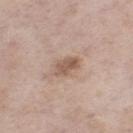{"biopsy_status": "not biopsied; imaged during a skin examination", "lighting": "white-light", "patient": {"sex": "female", "age_approx": 55}, "image": {"source": "total-body photography crop", "field_of_view_mm": 15}, "site": "left thigh", "automated_metrics": {"eccentricity": 0.85, "shape_asymmetry": 0.2, "cielab_L": 55, "cielab_a": 17, "cielab_b": 27, "vs_skin_darker_L": 11.0, "vs_skin_contrast_norm": 7.5, "border_irregularity_0_10": 2.0, "color_variation_0_10": 2.5, "peripheral_color_asymmetry": 1.0, "nevus_likeness_0_100": 15, "lesion_detection_confidence_0_100": 100}, "lesion_size": {"long_diameter_mm_approx": 3.5}}